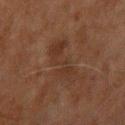biopsy status: total-body-photography surveillance lesion; no biopsy.